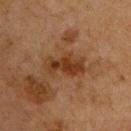Q: Was this lesion biopsied?
A: total-body-photography surveillance lesion; no biopsy
Q: Who is the patient?
A: male, in their mid- to late 70s
Q: Illumination type?
A: cross-polarized illumination
Q: How large is the lesion?
A: ≈5 mm
Q: Where on the body is the lesion?
A: the chest
Q: Automated lesion metrics?
A: a shape eccentricity near 0.85 and two-axis asymmetry of about 0.35; a mean CIELAB color near L≈29 a*≈18 b*≈28 and a lesion–skin lightness drop of about 8; internal color variation of about 3.5 on a 0–10 scale
Q: What is the imaging modality?
A: ~15 mm crop, total-body skin-cancer survey